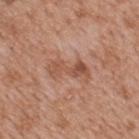Q: Was a biopsy performed?
A: imaged on a skin check; not biopsied
Q: Lesion location?
A: the back
Q: Patient demographics?
A: male, about 65 years old
Q: Lesion size?
A: about 5 mm
Q: How was this image acquired?
A: 15 mm crop, total-body photography
Q: Illumination type?
A: white-light illumination